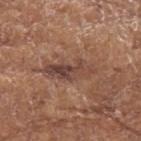Assessment: No biopsy was performed on this lesion — it was imaged during a full skin examination and was not determined to be concerning. Clinical summary: Cropped from a whole-body photographic skin survey; the tile spans about 15 mm. The lesion is located on the left forearm. Imaged with white-light lighting. A female subject in their mid- to late 70s. The lesion-visualizer software estimated an average lesion color of about L≈43 a*≈20 b*≈25 (CIELAB) and about 10 CIELAB-L* units darker than the surrounding skin. The analysis additionally found a classifier nevus-likeness of about 0/100 and a lesion-detection confidence of about 65/100. Measured at roughly 6 mm in maximum diameter.